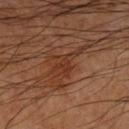biopsy status: catalogued during a skin exam; not biopsied
subject: male, aged approximately 60
site: the leg
lesion diameter: about 7.5 mm
acquisition: total-body-photography crop, ~15 mm field of view
automated metrics: a footprint of about 13 mm² and two-axis asymmetry of about 0.65; about 7 CIELAB-L* units darker than the surrounding skin; a border-irregularity index near 10/10, internal color variation of about 2.5 on a 0–10 scale, and radial color variation of about 1
tile lighting: cross-polarized